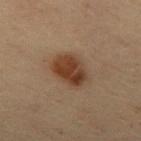image source: ~15 mm crop, total-body skin-cancer survey; lesion size: ~4.5 mm (longest diameter); patient: male, aged around 55; body site: the chest.